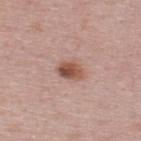workup: imaged on a skin check; not biopsied | diameter: ~3 mm (longest diameter) | image-analysis metrics: an average lesion color of about L≈52 a*≈22 b*≈28 (CIELAB), roughly 13 lightness units darker than nearby skin, and a lesion-to-skin contrast of about 9.5 (normalized; higher = more distinct); a nevus-likeness score of about 95/100 | image source: total-body-photography crop, ~15 mm field of view | patient: male, aged approximately 50 | anatomic site: the back | illumination: white-light illumination.